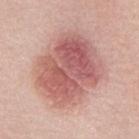No biopsy was performed on this lesion — it was imaged during a full skin examination and was not determined to be concerning. A female subject, about 50 years old. The tile uses white-light illumination. The lesion is on the upper back. A 15 mm crop from a total-body photograph taken for skin-cancer surveillance.